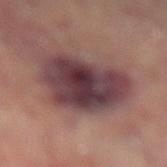Captured during whole-body skin photography for melanoma surveillance; the lesion was not biopsied. The subject is a male aged approximately 70. Measured at roughly 7 mm in maximum diameter. This image is a 15 mm lesion crop taken from a total-body photograph. The lesion is located on the left lower leg. An algorithmic analysis of the crop reported a footprint of about 31 mm², an eccentricity of roughly 0.55, and a shape-asymmetry score of about 0.25 (0 = symmetric). It also reported about 14 CIELAB-L* units darker than the surrounding skin and a normalized border contrast of about 13.5. The software also gave a color-variation rating of about 6.5/10 and radial color variation of about 2. The software also gave a detector confidence of about 100 out of 100 that the crop contains a lesion. The tile uses cross-polarized illumination.